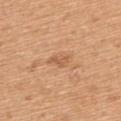Q: Was a biopsy performed?
A: imaged on a skin check; not biopsied
Q: What are the patient's age and sex?
A: female, roughly 55 years of age
Q: Where on the body is the lesion?
A: the upper back
Q: What is the imaging modality?
A: ~15 mm crop, total-body skin-cancer survey